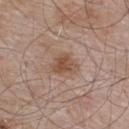Assessment:
Captured during whole-body skin photography for melanoma surveillance; the lesion was not biopsied.
Background:
Captured under white-light illumination. Approximately 3.5 mm at its widest. The total-body-photography lesion software estimated a footprint of about 6.5 mm², an eccentricity of roughly 0.65, and two-axis asymmetry of about 0.25. The software also gave an average lesion color of about L≈50 a*≈18 b*≈28 (CIELAB). It also reported an automated nevus-likeness rating near 75 out of 100 and a detector confidence of about 100 out of 100 that the crop contains a lesion. The patient is a male aged 53 to 57. The lesion is on the upper back. A 15 mm close-up tile from a total-body photography series done for melanoma screening.